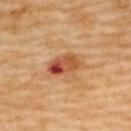Impression: No biopsy was performed on this lesion — it was imaged during a full skin examination and was not determined to be concerning. Background: The tile uses cross-polarized illumination. A 15 mm close-up extracted from a 3D total-body photography capture. An algorithmic analysis of the crop reported an automated nevus-likeness rating near 0 out of 100. A female patient aged 58–62. From the upper back.